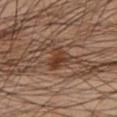The lesion was tiled from a total-body skin photograph and was not biopsied.
Captured under cross-polarized illumination.
Approximately 3.5 mm at its widest.
The lesion is located on the left lower leg.
Cropped from a whole-body photographic skin survey; the tile spans about 15 mm.
The total-body-photography lesion software estimated border irregularity of about 4 on a 0–10 scale, a color-variation rating of about 4/10, and radial color variation of about 1.5.
A male subject, roughly 50 years of age.